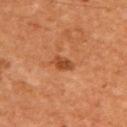The lesion was photographed on a routine skin check and not biopsied; there is no pathology result. An algorithmic analysis of the crop reported an area of roughly 4 mm², an outline eccentricity of about 0.85 (0 = round, 1 = elongated), and a symmetry-axis asymmetry near 0.35. It also reported border irregularity of about 3 on a 0–10 scale and a peripheral color-asymmetry measure near 0.5. It also reported a classifier nevus-likeness of about 30/100 and a detector confidence of about 100 out of 100 that the crop contains a lesion. The tile uses cross-polarized illumination. From the upper back. A 15 mm close-up tile from a total-body photography series done for melanoma screening. A male subject in their 60s.